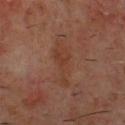Assessment:
Captured during whole-body skin photography for melanoma surveillance; the lesion was not biopsied.
Acquisition and patient details:
A male subject, about 60 years old. The lesion is on the chest. A lesion tile, about 15 mm wide, cut from a 3D total-body photograph. The total-body-photography lesion software estimated about 5 CIELAB-L* units darker than the surrounding skin and a normalized lesion–skin contrast near 5.5. It also reported an automated nevus-likeness rating near 0 out of 100 and a lesion-detection confidence of about 100/100.